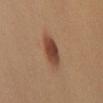{"biopsy_status": "not biopsied; imaged during a skin examination", "site": "left arm", "patient": {"sex": "female", "age_approx": 40}, "image": {"source": "total-body photography crop", "field_of_view_mm": 15}, "lighting": "cross-polarized"}